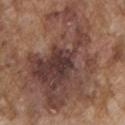Q: What kind of image is this?
A: 15 mm crop, total-body photography
Q: What did automated image analysis measure?
A: an area of roughly 75 mm², a shape eccentricity near 0.7, and a symmetry-axis asymmetry near 0.45; an average lesion color of about L≈42 a*≈19 b*≈23 (CIELAB), a lesion–skin lightness drop of about 11, and a normalized border contrast of about 9.5; a classifier nevus-likeness of about 10/100
Q: What are the patient's age and sex?
A: male, aged around 75
Q: Lesion location?
A: the chest
Q: How was the tile lit?
A: white-light